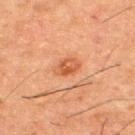{"biopsy_status": "not biopsied; imaged during a skin examination", "image": {"source": "total-body photography crop", "field_of_view_mm": 15}, "lighting": "cross-polarized", "site": "upper back", "automated_metrics": {"border_irregularity_0_10": 1.0, "color_variation_0_10": 4.0, "nevus_likeness_0_100": 80}, "patient": {"sex": "male", "age_approx": 50}, "lesion_size": {"long_diameter_mm_approx": 3.0}}